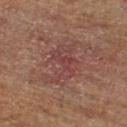workup: imaged on a skin check; not biopsied | patient: male, about 65 years old | body site: the right lower leg | illumination: cross-polarized illumination | automated lesion analysis: roughly 6 lightness units darker than nearby skin and a normalized lesion–skin contrast near 5.5; a nevus-likeness score of about 0/100 and a lesion-detection confidence of about 100/100 | diameter: ≈5.5 mm | image source: 15 mm crop, total-body photography.